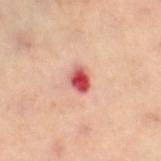Clinical impression: No biopsy was performed on this lesion — it was imaged during a full skin examination and was not determined to be concerning. Image and clinical context: This image is a 15 mm lesion crop taken from a total-body photograph. Located on the left leg. A female subject, roughly 60 years of age.